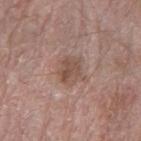Q: Was a biopsy performed?
A: no biopsy performed (imaged during a skin exam)
Q: What are the patient's age and sex?
A: male, aged 58 to 62
Q: Where on the body is the lesion?
A: the arm
Q: What is the imaging modality?
A: ~15 mm tile from a whole-body skin photo